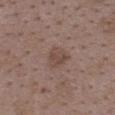<lesion>
<biopsy_status>not biopsied; imaged during a skin examination</biopsy_status>
<automated_metrics>
  <nevus_likeness_0_100>0</nevus_likeness_0_100>
  <lesion_detection_confidence_0_100>100</lesion_detection_confidence_0_100>
</automated_metrics>
<image>
  <source>total-body photography crop</source>
  <field_of_view_mm>15</field_of_view_mm>
</image>
<lesion_size>
  <long_diameter_mm_approx>3.5</long_diameter_mm_approx>
</lesion_size>
<site>upper back</site>
<patient>
  <sex>female</sex>
  <age_approx>35</age_approx>
</patient>
</lesion>